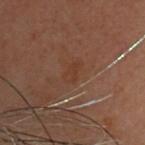imaging modality=~15 mm crop, total-body skin-cancer survey; lesion size=about 2.5 mm; patient=female, aged 58 to 62; location=the head or neck.